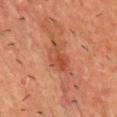patient:
  sex: male
  age_approx: 45
image:
  source: total-body photography crop
  field_of_view_mm: 15
site: chest